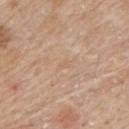Recorded during total-body skin imaging; not selected for excision or biopsy. The lesion-visualizer software estimated a lesion area of about 1 mm² and a shape-asymmetry score of about 0.25 (0 = symmetric). It also reported an average lesion color of about L≈62 a*≈17 b*≈32 (CIELAB), roughly 4 lightness units darker than nearby skin, and a normalized border contrast of about 3. It also reported border irregularity of about 2 on a 0–10 scale, a within-lesion color-variation index near 0/10, and peripheral color asymmetry of about 0. The subject is a male about 60 years old. A 15 mm close-up tile from a total-body photography series done for melanoma screening. Approximately 1 mm at its widest. Located on the mid back.